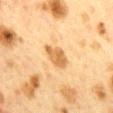– workup: imaged on a skin check; not biopsied
– imaging modality: ~15 mm crop, total-body skin-cancer survey
– location: the mid back
– subject: female, aged approximately 40